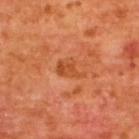Case summary:
– follow-up — total-body-photography surveillance lesion; no biopsy
– subject — male, aged 63–67
– tile lighting — cross-polarized
– imaging modality — 15 mm crop, total-body photography
– lesion size — ~3.5 mm (longest diameter)
– body site — the back
– automated metrics — a footprint of about 5 mm², an eccentricity of roughly 0.8, and a symmetry-axis asymmetry near 0.4; a mean CIELAB color near L≈49 a*≈30 b*≈42, about 7 CIELAB-L* units darker than the surrounding skin, and a normalized lesion–skin contrast near 6; a color-variation rating of about 2.5/10 and radial color variation of about 1; a classifier nevus-likeness of about 0/100 and a lesion-detection confidence of about 100/100